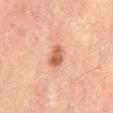biopsy status=imaged on a skin check; not biopsied | subject=male, in their 50s | lighting=cross-polarized | image source=~15 mm tile from a whole-body skin photo | site=the mid back | TBP lesion metrics=a lesion–skin lightness drop of about 10 and a normalized lesion–skin contrast near 8; a border-irregularity index near 2/10, internal color variation of about 3 on a 0–10 scale, and radial color variation of about 1; a lesion-detection confidence of about 100/100.